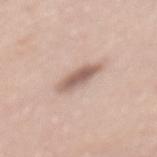{"biopsy_status": "not biopsied; imaged during a skin examination", "patient": {"sex": "female", "age_approx": 60}, "image": {"source": "total-body photography crop", "field_of_view_mm": 15}, "lighting": "white-light", "lesion_size": {"long_diameter_mm_approx": 4.5}, "site": "mid back"}